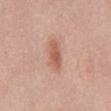No biopsy was performed on this lesion — it was imaged during a full skin examination and was not determined to be concerning. A female patient, aged 63–67. The lesion is on the front of the torso. Approximately 4 mm at its widest. The tile uses white-light illumination. Cropped from a total-body skin-imaging series; the visible field is about 15 mm.